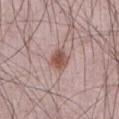Captured under white-light illumination. On the abdomen. This image is a 15 mm lesion crop taken from a total-body photograph. The patient is a male about 65 years old.